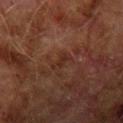follow-up: imaged on a skin check; not biopsied | image source: ~15 mm crop, total-body skin-cancer survey | body site: the left upper arm | lesion diameter: ~3 mm (longest diameter) | automated lesion analysis: a border-irregularity rating of about 5/10 and a peripheral color-asymmetry measure near 0 | patient: male, aged 73–77.